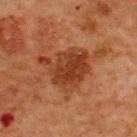Assessment: Imaged during a routine full-body skin examination; the lesion was not biopsied and no histopathology is available. Clinical summary: Automated image analysis of the tile measured a footprint of about 17 mm² and two-axis asymmetry of about 0.4. The analysis additionally found a mean CIELAB color near L≈34 a*≈25 b*≈33, roughly 9 lightness units darker than nearby skin, and a normalized lesion–skin contrast near 8.5. And it measured a border-irregularity rating of about 5/10, internal color variation of about 4 on a 0–10 scale, and peripheral color asymmetry of about 1.5. A male subject in their mid-60s. The lesion is on the upper back. Imaged with cross-polarized lighting. A 15 mm close-up extracted from a 3D total-body photography capture. Longest diameter approximately 6 mm.